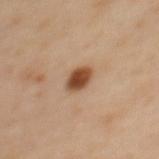Q: Was this lesion biopsied?
A: no biopsy performed (imaged during a skin exam)
Q: Who is the patient?
A: female, approximately 40 years of age
Q: Illumination type?
A: cross-polarized illumination
Q: Automated lesion metrics?
A: a lesion area of about 6 mm², an outline eccentricity of about 0.7 (0 = round, 1 = elongated), and two-axis asymmetry of about 0.2; an average lesion color of about L≈49 a*≈21 b*≈33 (CIELAB), roughly 15 lightness units darker than nearby skin, and a normalized lesion–skin contrast near 11; border irregularity of about 2 on a 0–10 scale, a color-variation rating of about 4.5/10, and radial color variation of about 1.5
Q: What is the imaging modality?
A: 15 mm crop, total-body photography
Q: How large is the lesion?
A: ~3 mm (longest diameter)
Q: Where on the body is the lesion?
A: the upper back